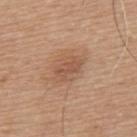Q: Was a biopsy performed?
A: catalogued during a skin exam; not biopsied
Q: What did automated image analysis measure?
A: about 8 CIELAB-L* units darker than the surrounding skin and a normalized border contrast of about 5.5; a border-irregularity index near 3/10, internal color variation of about 1.5 on a 0–10 scale, and radial color variation of about 0.5; an automated nevus-likeness rating near 30 out of 100 and a detector confidence of about 100 out of 100 that the crop contains a lesion
Q: Who is the patient?
A: male, aged approximately 65
Q: What is the lesion's diameter?
A: about 3.5 mm
Q: How was the tile lit?
A: white-light
Q: What is the imaging modality?
A: ~15 mm tile from a whole-body skin photo
Q: What is the anatomic site?
A: the upper back